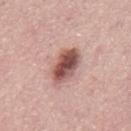Impression: Captured during whole-body skin photography for melanoma surveillance; the lesion was not biopsied. Context: A male patient aged 73–77. Located on the mid back. The recorded lesion diameter is about 5 mm. Cropped from a whole-body photographic skin survey; the tile spans about 15 mm. This is a white-light tile.